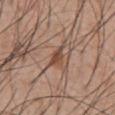{"biopsy_status": "not biopsied; imaged during a skin examination", "lighting": "white-light", "lesion_size": {"long_diameter_mm_approx": 2.5}, "patient": {"sex": "male", "age_approx": 55}, "image": {"source": "total-body photography crop", "field_of_view_mm": 15}, "site": "front of the torso"}